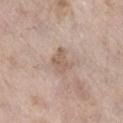Captured under white-light illumination. From the right lower leg. A roughly 15 mm field-of-view crop from a total-body skin photograph. The lesion's longest dimension is about 3 mm. The subject is a female roughly 75 years of age.